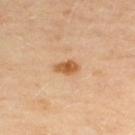Findings:
* follow-up — total-body-photography surveillance lesion; no biopsy
* subject — female, about 50 years old
* site — the chest
* image source — 15 mm crop, total-body photography